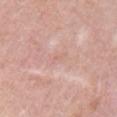<lesion>
  <lesion_size>
    <long_diameter_mm_approx>2.0</long_diameter_mm_approx>
  </lesion_size>
  <site>right upper arm</site>
  <patient>
    <sex>female</sex>
    <age_approx>50</age_approx>
  </patient>
  <image>
    <source>total-body photography crop</source>
    <field_of_view_mm>15</field_of_view_mm>
  </image>
</lesion>